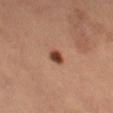Findings:
• biopsy status · no biopsy performed (imaged during a skin exam)
• patient · female, aged around 65
• body site · the left thigh
• image · ~15 mm tile from a whole-body skin photo